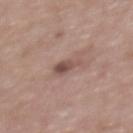Impression: Part of a total-body skin-imaging series; this lesion was reviewed on a skin check and was not flagged for biopsy. Image and clinical context: Automated tile analysis of the lesion measured a lesion area of about 4.5 mm². The analysis additionally found an average lesion color of about L≈50 a*≈19 b*≈22 (CIELAB) and a lesion-to-skin contrast of about 7.5 (normalized; higher = more distinct). And it measured a border-irregularity index near 4/10, a within-lesion color-variation index near 3.5/10, and radial color variation of about 1.5. And it measured an automated nevus-likeness rating near 10 out of 100 and lesion-presence confidence of about 100/100. A female subject aged 48 to 52. A 15 mm crop from a total-body photograph taken for skin-cancer surveillance. The lesion is on the upper back. The lesion's longest dimension is about 3.5 mm.